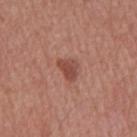Recorded during total-body skin imaging; not selected for excision or biopsy. Measured at roughly 3 mm in maximum diameter. Captured under white-light illumination. From the chest. A male patient in their mid-40s. Cropped from a whole-body photographic skin survey; the tile spans about 15 mm.